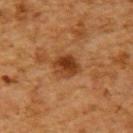Q: How was this image acquired?
A: total-body-photography crop, ~15 mm field of view
Q: Lesion size?
A: about 3 mm
Q: Who is the patient?
A: male, roughly 60 years of age
Q: How was the tile lit?
A: cross-polarized illumination
Q: Where on the body is the lesion?
A: the left upper arm